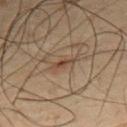Recorded during total-body skin imaging; not selected for excision or biopsy. A roughly 15 mm field-of-view crop from a total-body skin photograph. The lesion's longest dimension is about 2.5 mm. A male patient aged approximately 50. This is a cross-polarized tile. From the chest.